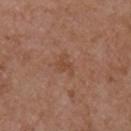The lesion was photographed on a routine skin check and not biopsied; there is no pathology result. About 2.5 mm across. A male patient, aged 53–57. A lesion tile, about 15 mm wide, cut from a 3D total-body photograph. On the chest. Imaged with white-light lighting.